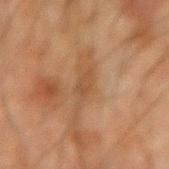No biopsy was performed on this lesion — it was imaged during a full skin examination and was not determined to be concerning. About 4 mm across. Captured under cross-polarized illumination. Cropped from a whole-body photographic skin survey; the tile spans about 15 mm. The subject is a male aged 63 to 67. On the right forearm.